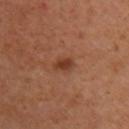Impression: This lesion was catalogued during total-body skin photography and was not selected for biopsy. Acquisition and patient details: A female patient aged 38 to 42. Imaged with cross-polarized lighting. The lesion's longest dimension is about 2.5 mm. Cropped from a whole-body photographic skin survey; the tile spans about 15 mm. The lesion is on the right upper arm.